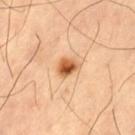Findings:
• automated metrics · a footprint of about 5.5 mm², an outline eccentricity of about 0.55 (0 = round, 1 = elongated), and a shape-asymmetry score of about 0.3 (0 = symmetric); a color-variation rating of about 8.5/10 and peripheral color asymmetry of about 3; a classifier nevus-likeness of about 100/100
• diameter · ≈3 mm
• imaging modality · 15 mm crop, total-body photography
• lighting · cross-polarized illumination
• location · the left thigh
• subject · male, roughly 60 years of age